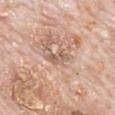Part of a total-body skin-imaging series; this lesion was reviewed on a skin check and was not flagged for biopsy. Located on the mid back. About 3 mm across. A male subject in their 80s. A 15 mm close-up tile from a total-body photography series done for melanoma screening.